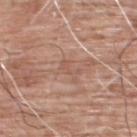<lesion>
  <biopsy_status>not biopsied; imaged during a skin examination</biopsy_status>
  <image>
    <source>total-body photography crop</source>
    <field_of_view_mm>15</field_of_view_mm>
  </image>
  <site>upper back</site>
  <patient>
    <sex>male</sex>
    <age_approx>60</age_approx>
  </patient>
  <lesion_size>
    <long_diameter_mm_approx>2.5</long_diameter_mm_approx>
  </lesion_size>
  <lighting>white-light</lighting>
  <automated_metrics>
    <cielab_L>55</cielab_L>
    <cielab_a>20</cielab_a>
    <cielab_b>28</cielab_b>
    <vs_skin_darker_L>6.0</vs_skin_darker_L>
    <vs_skin_contrast_norm>4.5</vs_skin_contrast_norm>
  </automated_metrics>
</lesion>